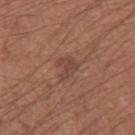Q: Was a biopsy performed?
A: total-body-photography surveillance lesion; no biopsy
Q: Where on the body is the lesion?
A: the left upper arm
Q: Illumination type?
A: white-light illumination
Q: What kind of image is this?
A: ~15 mm crop, total-body skin-cancer survey
Q: Lesion size?
A: ≈3 mm
Q: Automated lesion metrics?
A: a lesion area of about 4.5 mm², an outline eccentricity of about 0.65 (0 = round, 1 = elongated), and a shape-asymmetry score of about 0.45 (0 = symmetric); an average lesion color of about L≈44 a*≈21 b*≈25 (CIELAB) and a normalized lesion–skin contrast near 6; a classifier nevus-likeness of about 0/100
Q: Who is the patient?
A: male, aged 33–37